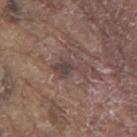Assessment:
No biopsy was performed on this lesion — it was imaged during a full skin examination and was not determined to be concerning.
Context:
The recorded lesion diameter is about 4 mm. The lesion is located on the mid back. A male subject aged 78–82. Cropped from a total-body skin-imaging series; the visible field is about 15 mm.